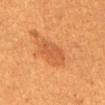{
  "biopsy_status": "not biopsied; imaged during a skin examination",
  "image": {
    "source": "total-body photography crop",
    "field_of_view_mm": 15
  },
  "lesion_size": {
    "long_diameter_mm_approx": 3.5
  },
  "patient": {
    "sex": "female",
    "age_approx": 55
  },
  "site": "abdomen",
  "automated_metrics": {
    "area_mm2_approx": 7.5,
    "eccentricity": 0.7,
    "shape_asymmetry": 0.25,
    "cielab_L": 48,
    "cielab_a": 26,
    "cielab_b": 37,
    "vs_skin_darker_L": 7.0,
    "vs_skin_contrast_norm": 5.0,
    "peripheral_color_asymmetry": 1.0,
    "nevus_likeness_0_100": 70,
    "lesion_detection_confidence_0_100": 100
  },
  "lighting": "cross-polarized"
}